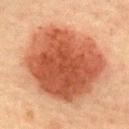{
  "biopsy_status": "not biopsied; imaged during a skin examination",
  "patient": {
    "sex": "male",
    "age_approx": 60
  },
  "image": {
    "source": "total-body photography crop",
    "field_of_view_mm": 15
  },
  "lesion_size": {
    "long_diameter_mm_approx": 10.5
  },
  "lighting": "cross-polarized",
  "site": "back"
}